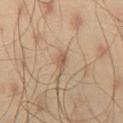biopsy status: catalogued during a skin exam; not biopsied | anatomic site: the right thigh | TBP lesion metrics: an area of roughly 2.5 mm² and a symmetry-axis asymmetry near 0.35; a mean CIELAB color near L≈55 a*≈17 b*≈30 and a normalized lesion–skin contrast near 6 | subject: male, aged around 45 | image source: ~15 mm tile from a whole-body skin photo | lesion diameter: about 2.5 mm.